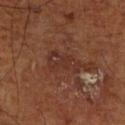Assessment:
Part of a total-body skin-imaging series; this lesion was reviewed on a skin check and was not flagged for biopsy.
Acquisition and patient details:
The patient is a male aged 68–72. Cropped from a total-body skin-imaging series; the visible field is about 15 mm. The lesion is located on the right lower leg. About 4 mm across. Captured under cross-polarized illumination. Automated tile analysis of the lesion measured roughly 6 lightness units darker than nearby skin. The analysis additionally found a border-irregularity index near 4/10, internal color variation of about 3.5 on a 0–10 scale, and a peripheral color-asymmetry measure near 1. And it measured a nevus-likeness score of about 0/100 and a lesion-detection confidence of about 75/100.